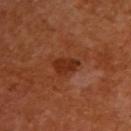The lesion was photographed on a routine skin check and not biopsied; there is no pathology result.
About 3.5 mm across.
An algorithmic analysis of the crop reported a mean CIELAB color near L≈33 a*≈27 b*≈34, a lesion–skin lightness drop of about 9, and a normalized border contrast of about 8. It also reported a border-irregularity index near 3.5/10, a color-variation rating of about 2/10, and a peripheral color-asymmetry measure near 1. It also reported a classifier nevus-likeness of about 10/100 and lesion-presence confidence of about 100/100.
The subject is a female aged 53 to 57.
A lesion tile, about 15 mm wide, cut from a 3D total-body photograph.
From the upper back.
Captured under cross-polarized illumination.